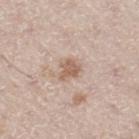* workup: no biopsy performed (imaged during a skin exam)
* imaging modality: ~15 mm tile from a whole-body skin photo
* patient: male, in their mid- to late 60s
* lesion diameter: ~2.5 mm (longest diameter)
* illumination: white-light illumination
* location: the right thigh